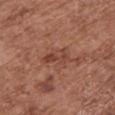follow-up: no biopsy performed (imaged during a skin exam)
location: the chest
patient: female, in their mid-70s
lesion size: ≈3.5 mm
illumination: white-light illumination
imaging modality: ~15 mm crop, total-body skin-cancer survey
TBP lesion metrics: a lesion color around L≈43 a*≈23 b*≈27 in CIELAB, roughly 8 lightness units darker than nearby skin, and a normalized border contrast of about 6; a border-irregularity rating of about 3/10, internal color variation of about 4.5 on a 0–10 scale, and radial color variation of about 1.5; an automated nevus-likeness rating near 0 out of 100 and a detector confidence of about 100 out of 100 that the crop contains a lesion